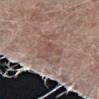Clinical impression: Recorded during total-body skin imaging; not selected for excision or biopsy. Acquisition and patient details: A male subject roughly 80 years of age. This is a white-light tile. A 15 mm close-up tile from a total-body photography series done for melanoma screening. The total-body-photography lesion software estimated an area of roughly 10 mm² and a symmetry-axis asymmetry near 0.55. It also reported a mean CIELAB color near L≈51 a*≈17 b*≈22. It also reported border irregularity of about 9 on a 0–10 scale, a color-variation rating of about 2.5/10, and a peripheral color-asymmetry measure near 0.5. The analysis additionally found an automated nevus-likeness rating near 0 out of 100 and a lesion-detection confidence of about 80/100. The lesion is located on the left forearm.